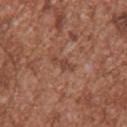Imaged during a routine full-body skin examination; the lesion was not biopsied and no histopathology is available. Cropped from a whole-body photographic skin survey; the tile spans about 15 mm. Located on the upper back. The lesion-visualizer software estimated an area of roughly 3 mm², an eccentricity of roughly 0.95, and a symmetry-axis asymmetry near 0.25. About 3 mm across. The subject is a male aged approximately 45. Imaged with white-light lighting.